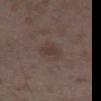Acquisition and patient details:
A roughly 15 mm field-of-view crop from a total-body skin photograph. The recorded lesion diameter is about 3 mm. On the right lower leg. A female patient, in their mid-30s. Imaged with white-light lighting. Automated tile analysis of the lesion measured a lesion area of about 5 mm² and a shape-asymmetry score of about 0.35 (0 = symmetric). And it measured border irregularity of about 3.5 on a 0–10 scale, a within-lesion color-variation index near 1.5/10, and radial color variation of about 0.5.